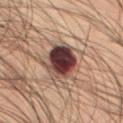Impression:
The lesion was photographed on a routine skin check and not biopsied; there is no pathology result.
Context:
The lesion's longest dimension is about 4.5 mm. The total-body-photography lesion software estimated a border-irregularity rating of about 1.5/10, a within-lesion color-variation index near 7.5/10, and radial color variation of about 2. The analysis additionally found a classifier nevus-likeness of about 90/100 and lesion-presence confidence of about 100/100. Located on the left thigh. A 15 mm close-up tile from a total-body photography series done for melanoma screening. This is a cross-polarized tile. The subject is a male aged around 50.